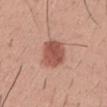Assessment:
Part of a total-body skin-imaging series; this lesion was reviewed on a skin check and was not flagged for biopsy.
Background:
Captured under white-light illumination. A lesion tile, about 15 mm wide, cut from a 3D total-body photograph. Located on the mid back. Automated tile analysis of the lesion measured an area of roughly 11 mm². The software also gave border irregularity of about 2 on a 0–10 scale, a color-variation rating of about 3.5/10, and a peripheral color-asymmetry measure near 1. The subject is a male aged approximately 30. About 4.5 mm across.